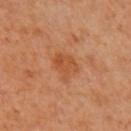This lesion was catalogued during total-body skin photography and was not selected for biopsy.
A roughly 15 mm field-of-view crop from a total-body skin photograph.
About 3.5 mm across.
The patient is female.
Located on the arm.